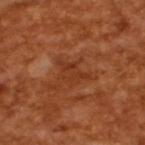• workup · total-body-photography surveillance lesion; no biopsy
• imaging modality · 15 mm crop, total-body photography
• patient · male, about 65 years old
• size · about 4 mm
• lighting · cross-polarized